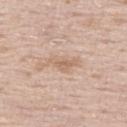Background: A male patient, aged 63 to 67. This is a white-light tile. A 15 mm close-up extracted from a 3D total-body photography capture. On the left thigh. The total-body-photography lesion software estimated an area of roughly 4.5 mm² and an outline eccentricity of about 0.9 (0 = round, 1 = elongated). And it measured roughly 8 lightness units darker than nearby skin and a normalized border contrast of about 6. And it measured an automated nevus-likeness rating near 0 out of 100 and a lesion-detection confidence of about 100/100. Approximately 3.5 mm at its widest.